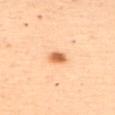biopsy status: imaged on a skin check; not biopsied | illumination: cross-polarized illumination | imaging modality: ~15 mm tile from a whole-body skin photo | site: the upper back | image-analysis metrics: a footprint of about 3.5 mm², a shape eccentricity near 0.75, and a symmetry-axis asymmetry near 0.2; an average lesion color of about L≈57 a*≈23 b*≈37 (CIELAB), a lesion–skin lightness drop of about 14, and a lesion-to-skin contrast of about 9 (normalized; higher = more distinct); an automated nevus-likeness rating near 100 out of 100 | patient: female, aged around 50.